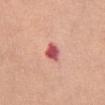An algorithmic analysis of the crop reported about 17 CIELAB-L* units darker than the surrounding skin.
A female patient aged 63 to 67.
Longest diameter approximately 2.5 mm.
Captured under white-light illumination.
On the right thigh.
Cropped from a whole-body photographic skin survey; the tile spans about 15 mm.